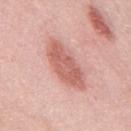notes=imaged on a skin check; not biopsied | image=total-body-photography crop, ~15 mm field of view | lighting=white-light | diameter=about 7 mm | image-analysis metrics=a footprint of about 15 mm²; a border-irregularity rating of about 2.5/10, a within-lesion color-variation index near 3/10, and radial color variation of about 1; a detector confidence of about 100 out of 100 that the crop contains a lesion | site=the mid back | patient=male, about 55 years old.